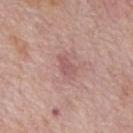biopsy status = total-body-photography surveillance lesion; no biopsy
body site = the mid back
tile lighting = white-light
automated metrics = a footprint of about 3.5 mm², an eccentricity of roughly 0.85, and two-axis asymmetry of about 0.4
lesion diameter = ~3 mm (longest diameter)
subject = male, approximately 75 years of age
image = ~15 mm tile from a whole-body skin photo